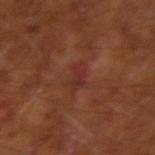Assessment: The lesion was tiled from a total-body skin photograph and was not biopsied. Acquisition and patient details: A 15 mm close-up extracted from a 3D total-body photography capture. Measured at roughly 3 mm in maximum diameter. The lesion is located on the arm. The subject is a male aged around 65.